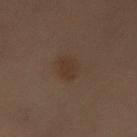tile lighting=cross-polarized; location=the right leg; imaging modality=total-body-photography crop, ~15 mm field of view; subject=female, approximately 60 years of age.